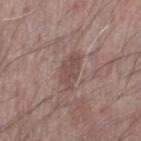Q: How was this image acquired?
A: 15 mm crop, total-body photography
Q: Who is the patient?
A: male, aged 68 to 72
Q: What is the anatomic site?
A: the left forearm
Q: What is the lesion's diameter?
A: ≈4 mm